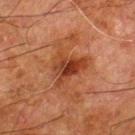The lesion was photographed on a routine skin check and not biopsied; there is no pathology result. The recorded lesion diameter is about 8 mm. Cropped from a total-body skin-imaging series; the visible field is about 15 mm. This is a cross-polarized tile. The subject is a male in their 80s. The lesion is located on the left thigh.